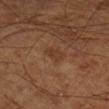{"biopsy_status": "not biopsied; imaged during a skin examination", "patient": {"age_approx": 65}, "lesion_size": {"long_diameter_mm_approx": 2.5}, "automated_metrics": {"border_irregularity_0_10": 3.0, "color_variation_0_10": 1.0}, "image": {"source": "total-body photography crop", "field_of_view_mm": 15}, "site": "right lower leg", "lighting": "cross-polarized"}